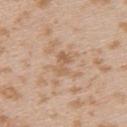Q: Was this lesion biopsied?
A: total-body-photography surveillance lesion; no biopsy
Q: Automated lesion metrics?
A: an area of roughly 3.5 mm² and two-axis asymmetry of about 0.35; a lesion–skin lightness drop of about 7; border irregularity of about 4.5 on a 0–10 scale and a color-variation rating of about 1/10; an automated nevus-likeness rating near 0 out of 100
Q: How was this image acquired?
A: ~15 mm tile from a whole-body skin photo
Q: How large is the lesion?
A: ≈2.5 mm
Q: Patient demographics?
A: female, in their mid-20s
Q: What is the anatomic site?
A: the upper back